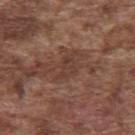Q: Was a biopsy performed?
A: imaged on a skin check; not biopsied
Q: What are the patient's age and sex?
A: male, aged 73 to 77
Q: How was this image acquired?
A: ~15 mm tile from a whole-body skin photo
Q: Lesion location?
A: the upper back